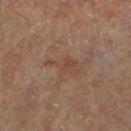<record>
<biopsy_status>not biopsied; imaged during a skin examination</biopsy_status>
<patient>
  <sex>male</sex>
  <age_approx>85+</age_approx>
</patient>
<automated_metrics>
  <area_mm2_approx>5.0</area_mm2_approx>
  <eccentricity>0.9</eccentricity>
  <vs_skin_darker_L>5.0</vs_skin_darker_L>
  <vs_skin_contrast_norm>5.5</vs_skin_contrast_norm>
  <nevus_likeness_0_100>0</nevus_likeness_0_100>
</automated_metrics>
<image>
  <source>total-body photography crop</source>
  <field_of_view_mm>15</field_of_view_mm>
</image>
<site>left lower leg</site>
<lesion_size>
  <long_diameter_mm_approx>4.0</long_diameter_mm_approx>
</lesion_size>
</record>